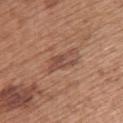Case summary:
- workup · catalogued during a skin exam; not biopsied
- subject · female, roughly 65 years of age
- imaging modality · total-body-photography crop, ~15 mm field of view
- body site · the back
- illumination · white-light